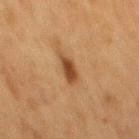Impression: Captured during whole-body skin photography for melanoma surveillance; the lesion was not biopsied. Context: The lesion is located on the mid back. A close-up tile cropped from a whole-body skin photograph, about 15 mm across. This is a cross-polarized tile. Measured at roughly 3.5 mm in maximum diameter. The total-body-photography lesion software estimated a footprint of about 5 mm², a shape eccentricity near 0.85, and a symmetry-axis asymmetry near 0.2. A male subject, aged around 75.